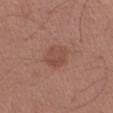Clinical impression: This lesion was catalogued during total-body skin photography and was not selected for biopsy. Acquisition and patient details: The subject is a male in their mid- to late 40s. Longest diameter approximately 3.5 mm. Cropped from a whole-body photographic skin survey; the tile spans about 15 mm. From the left forearm.